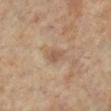Imaged during a routine full-body skin examination; the lesion was not biopsied and no histopathology is available.
A female patient roughly 65 years of age.
Located on the right leg.
A 15 mm close-up extracted from a 3D total-body photography capture.
Measured at roughly 2.5 mm in maximum diameter.